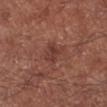No biopsy was performed on this lesion — it was imaged during a full skin examination and was not determined to be concerning. The subject is a male about 70 years old. A 15 mm close-up tile from a total-body photography series done for melanoma screening. Located on the right lower leg.